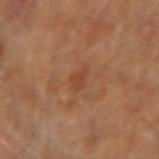{"biopsy_status": "not biopsied; imaged during a skin examination", "image": {"source": "total-body photography crop", "field_of_view_mm": 15}, "automated_metrics": {"vs_skin_darker_L": 6.0, "nevus_likeness_0_100": 0}, "lesion_size": {"long_diameter_mm_approx": 3.0}, "lighting": "cross-polarized", "patient": {"sex": "male", "age_approx": 65}, "site": "right upper arm"}